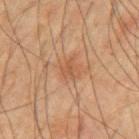Notes:
* workup — no biopsy performed (imaged during a skin exam)
* subject — male, in their mid- to late 60s
* anatomic site — the left upper arm
* acquisition — ~15 mm crop, total-body skin-cancer survey
* lighting — cross-polarized illumination
* size — ~2.5 mm (longest diameter)